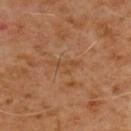Notes:
• follow-up: no biopsy performed (imaged during a skin exam)
• acquisition: ~15 mm tile from a whole-body skin photo
• patient: male, aged 58–62
• illumination: cross-polarized illumination
• lesion diameter: ≈3.5 mm
• automated metrics: border irregularity of about 8 on a 0–10 scale, a color-variation rating of about 0/10, and peripheral color asymmetry of about 0
• site: the chest